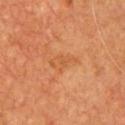Impression: This lesion was catalogued during total-body skin photography and was not selected for biopsy. Acquisition and patient details: The subject is a male about 60 years old. The lesion's longest dimension is about 3.5 mm. This image is a 15 mm lesion crop taken from a total-body photograph. This is a cross-polarized tile. On the chest.